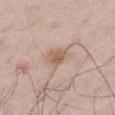This lesion was catalogued during total-body skin photography and was not selected for biopsy. The tile uses white-light illumination. A male patient, in their mid- to late 60s. About 3 mm across. From the right thigh. Cropped from a total-body skin-imaging series; the visible field is about 15 mm.